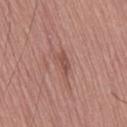The lesion was photographed on a routine skin check and not biopsied; there is no pathology result.
Automated image analysis of the tile measured a footprint of about 4 mm², an outline eccentricity of about 0.85 (0 = round, 1 = elongated), and a symmetry-axis asymmetry near 0.35. The software also gave an average lesion color of about L≈51 a*≈23 b*≈26 (CIELAB) and a normalized border contrast of about 6.
The tile uses white-light illumination.
Measured at roughly 3 mm in maximum diameter.
Cropped from a total-body skin-imaging series; the visible field is about 15 mm.
On the leg.
The patient is a male about 75 years old.